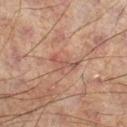Recorded during total-body skin imaging; not selected for excision or biopsy. Imaged with cross-polarized lighting. Cropped from a whole-body photographic skin survey; the tile spans about 15 mm. Measured at roughly 3.5 mm in maximum diameter. Automated tile analysis of the lesion measured an eccentricity of roughly 0.95. The software also gave border irregularity of about 6.5 on a 0–10 scale, a within-lesion color-variation index near 0/10, and a peripheral color-asymmetry measure near 0. Located on the left leg. A male patient, roughly 60 years of age.